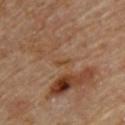{
  "biopsy_status": "not biopsied; imaged during a skin examination",
  "lesion_size": {
    "long_diameter_mm_approx": 2.0
  },
  "patient": {
    "sex": "male",
    "age_approx": "85+"
  },
  "lighting": "cross-polarized",
  "site": "back",
  "automated_metrics": {
    "area_mm2_approx": 1.0,
    "eccentricity": 0.95,
    "shape_asymmetry": 0.45,
    "nevus_likeness_0_100": 0,
    "lesion_detection_confidence_0_100": 90
  },
  "image": {
    "source": "total-body photography crop",
    "field_of_view_mm": 15
  }
}